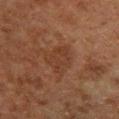workup: catalogued during a skin exam; not biopsied
patient: male, approximately 80 years of age
image: 15 mm crop, total-body photography
body site: the left lower leg